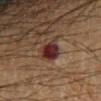Case summary:
– biopsy status: no biopsy performed (imaged during a skin exam)
– anatomic site: the right forearm
– patient: male, about 60 years old
– lighting: cross-polarized illumination
– imaging modality: total-body-photography crop, ~15 mm field of view